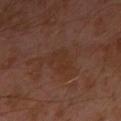Context:
The subject is a male aged approximately 30. A 15 mm close-up tile from a total-body photography series done for melanoma screening. The lesion is on the left arm. Longest diameter approximately 3.5 mm. Imaged with cross-polarized lighting.